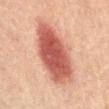workup: total-body-photography surveillance lesion; no biopsy
body site: the abdomen
lesion size: about 9.5 mm
lighting: cross-polarized illumination
image: total-body-photography crop, ~15 mm field of view
subject: male, aged 58 to 62
automated metrics: an area of roughly 30 mm²